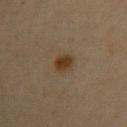Recorded during total-body skin imaging; not selected for excision or biopsy. The total-body-photography lesion software estimated a mean CIELAB color near L≈31 a*≈12 b*≈27, roughly 8 lightness units darker than nearby skin, and a normalized border contrast of about 9.5. And it measured a nevus-likeness score of about 95/100 and a lesion-detection confidence of about 100/100. A 15 mm close-up extracted from a 3D total-body photography capture. A female patient, aged 43–47. From the left upper arm. About 2.5 mm across. Captured under cross-polarized illumination.